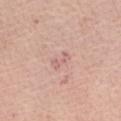The lesion was photographed on a routine skin check and not biopsied; there is no pathology result.